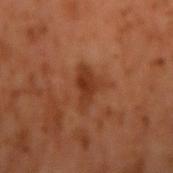Q: Automated lesion metrics?
A: about 9 CIELAB-L* units darker than the surrounding skin and a normalized lesion–skin contrast near 8; border irregularity of about 5 on a 0–10 scale
Q: What is the lesion's diameter?
A: about 4 mm
Q: What is the imaging modality?
A: ~15 mm tile from a whole-body skin photo
Q: Patient demographics?
A: male, aged 58 to 62
Q: Illumination type?
A: cross-polarized
Q: What is the anatomic site?
A: the arm